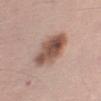Background:
A 15 mm close-up extracted from a 3D total-body photography capture. The lesion is located on the left upper arm. The tile uses white-light illumination. A male patient aged 68 to 72.